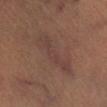lighting: cross-polarized illumination | image: ~15 mm crop, total-body skin-cancer survey | automated metrics: a footprint of about 10 mm², a shape eccentricity near 0.95, and a shape-asymmetry score of about 0.4 (0 = symmetric); a border-irregularity index near 6/10, internal color variation of about 3 on a 0–10 scale, and peripheral color asymmetry of about 1 | subject: male, aged 48–52 | site: the right lower leg.